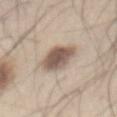Findings:
- workup: no biopsy performed (imaged during a skin exam)
- image: ~15 mm tile from a whole-body skin photo
- subject: male, in their mid-40s
- location: the abdomen
- illumination: white-light illumination
- lesion diameter: about 4 mm